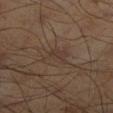<case>
  <lighting>cross-polarized</lighting>
  <site>right lower leg</site>
  <patient>
    <sex>male</sex>
    <age_approx>45</age_approx>
  </patient>
  <lesion_size>
    <long_diameter_mm_approx>3.5</long_diameter_mm_approx>
  </lesion_size>
  <image>
    <source>total-body photography crop</source>
    <field_of_view_mm>15</field_of_view_mm>
  </image>
</case>